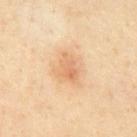Findings:
– biopsy status: no biopsy performed (imaged during a skin exam)
– imaging modality: 15 mm crop, total-body photography
– patient: male, approximately 55 years of age
– body site: the abdomen